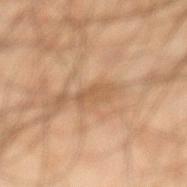Imaged during a routine full-body skin examination; the lesion was not biopsied and no histopathology is available. On the left lower leg. The patient is a male aged approximately 45. The lesion's longest dimension is about 3 mm. This image is a 15 mm lesion crop taken from a total-body photograph. Imaged with cross-polarized lighting.